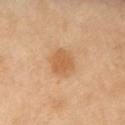From the chest. A close-up tile cropped from a whole-body skin photograph, about 15 mm across. A female patient, about 50 years old.